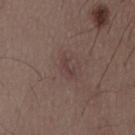Clinical impression: Captured during whole-body skin photography for melanoma surveillance; the lesion was not biopsied. Background: The patient is a male roughly 50 years of age. Cropped from a total-body skin-imaging series; the visible field is about 15 mm. The lesion is on the lower back. The tile uses white-light illumination. Longest diameter approximately 3 mm. An algorithmic analysis of the crop reported a nevus-likeness score of about 0/100.